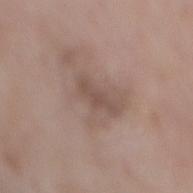Clinical impression:
The lesion was tiled from a total-body skin photograph and was not biopsied.
Image and clinical context:
A close-up tile cropped from a whole-body skin photograph, about 15 mm across. Longest diameter approximately 4 mm. On the back. The patient is a female in their 70s. Captured under white-light illumination.